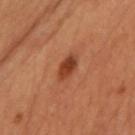{
  "biopsy_status": "not biopsied; imaged during a skin examination",
  "patient": {
    "sex": "female",
    "age_approx": 50
  },
  "site": "chest",
  "automated_metrics": {
    "cielab_L": 35,
    "cielab_a": 24,
    "cielab_b": 31,
    "vs_skin_darker_L": 10.0,
    "vs_skin_contrast_norm": 9.0,
    "color_variation_0_10": 3.5,
    "peripheral_color_asymmetry": 1.0,
    "nevus_likeness_0_100": 95,
    "lesion_detection_confidence_0_100": 100
  },
  "lighting": "cross-polarized",
  "lesion_size": {
    "long_diameter_mm_approx": 3.5
  },
  "image": {
    "source": "total-body photography crop",
    "field_of_view_mm": 15
  }
}